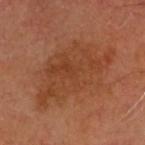Q: What is the anatomic site?
A: the head or neck
Q: What lighting was used for the tile?
A: cross-polarized illumination
Q: What are the patient's age and sex?
A: approximately 65 years of age
Q: How was this image acquired?
A: total-body-photography crop, ~15 mm field of view